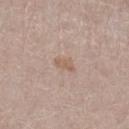Clinical impression:
Part of a total-body skin-imaging series; this lesion was reviewed on a skin check and was not flagged for biopsy.
Clinical summary:
The lesion is located on the right lower leg. Captured under white-light illumination. A female subject in their 40s. Automated tile analysis of the lesion measured border irregularity of about 3.5 on a 0–10 scale and peripheral color asymmetry of about 0. It also reported an automated nevus-likeness rating near 0 out of 100 and lesion-presence confidence of about 100/100. A close-up tile cropped from a whole-body skin photograph, about 15 mm across.